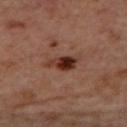Assessment: Imaged during a routine full-body skin examination; the lesion was not biopsied and no histopathology is available. Acquisition and patient details: A female subject aged around 45. The lesion is located on the back. An algorithmic analysis of the crop reported a footprint of about 5.5 mm², an outline eccentricity of about 0.9 (0 = round, 1 = elongated), and a symmetry-axis asymmetry near 0.3. It also reported a lesion color around L≈31 a*≈22 b*≈27 in CIELAB and a lesion–skin lightness drop of about 13. The software also gave a border-irregularity index near 3.5/10, a within-lesion color-variation index near 6/10, and radial color variation of about 2. And it measured a classifier nevus-likeness of about 95/100 and a detector confidence of about 100 out of 100 that the crop contains a lesion. Measured at roughly 4 mm in maximum diameter. A region of skin cropped from a whole-body photographic capture, roughly 15 mm wide. The tile uses cross-polarized illumination.